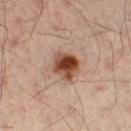The lesion was tiled from a total-body skin photograph and was not biopsied. From the left leg. A region of skin cropped from a whole-body photographic capture, roughly 15 mm wide. A male subject about 50 years old. The lesion-visualizer software estimated a border-irregularity rating of about 1.5/10, a within-lesion color-variation index near 9.5/10, and a peripheral color-asymmetry measure near 3. The software also gave an automated nevus-likeness rating near 100 out of 100 and lesion-presence confidence of about 100/100. The recorded lesion diameter is about 3.5 mm. This is a cross-polarized tile.